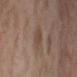image source=total-body-photography crop, ~15 mm field of view | illumination=cross-polarized illumination | subject=female, approximately 55 years of age | diameter=≈3 mm | anatomic site=the leg.